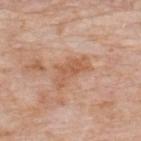{
  "automated_metrics": {
    "area_mm2_approx": 8.5,
    "shape_asymmetry": 0.4,
    "border_irregularity_0_10": 5.0,
    "color_variation_0_10": 3.0,
    "peripheral_color_asymmetry": 1.0,
    "nevus_likeness_0_100": 0,
    "lesion_detection_confidence_0_100": 100
  },
  "site": "upper back",
  "lesion_size": {
    "long_diameter_mm_approx": 4.5
  },
  "patient": {
    "sex": "male",
    "age_approx": 75
  },
  "image": {
    "source": "total-body photography crop",
    "field_of_view_mm": 15
  },
  "lighting": "white-light"
}